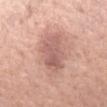Notes:
– follow-up: no biopsy performed (imaged during a skin exam)
– subject: female, aged 58–62
– image: ~15 mm crop, total-body skin-cancer survey
– tile lighting: white-light illumination
– diameter: ~5 mm (longest diameter)
– anatomic site: the left forearm